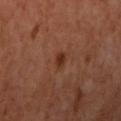anatomic site=the right upper arm
tile lighting=cross-polarized
diameter=about 2.5 mm
automated lesion analysis=an average lesion color of about L≈34 a*≈24 b*≈31 (CIELAB); a nevus-likeness score of about 90/100 and a detector confidence of about 100 out of 100 that the crop contains a lesion
patient=female, in their 50s
image source=total-body-photography crop, ~15 mm field of view